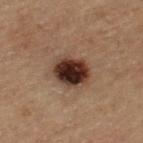Cropped from a total-body skin-imaging series; the visible field is about 15 mm. A male patient, approximately 70 years of age. On the left thigh.